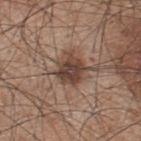Clinical impression: This lesion was catalogued during total-body skin photography and was not selected for biopsy. Context: The lesion's longest dimension is about 3.5 mm. From the upper back. A male patient in their mid- to late 40s. Captured under white-light illumination. A close-up tile cropped from a whole-body skin photograph, about 15 mm across.